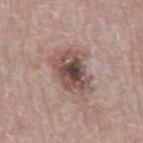Background: A lesion tile, about 15 mm wide, cut from a 3D total-body photograph. The lesion is on the abdomen. The subject is a male aged around 70.